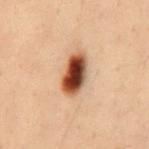The lesion is located on the mid back. Imaged with cross-polarized lighting. The subject is a male aged 28–32. A lesion tile, about 15 mm wide, cut from a 3D total-body photograph. Measured at roughly 5 mm in maximum diameter.